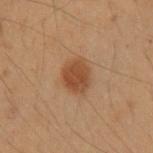<lesion>
<biopsy_status>not biopsied; imaged during a skin examination</biopsy_status>
<lighting>cross-polarized</lighting>
<automated_metrics>
  <area_mm2_approx>9.0</area_mm2_approx>
  <shape_asymmetry>0.15</shape_asymmetry>
  <cielab_L>38</cielab_L>
  <cielab_a>18</cielab_a>
  <cielab_b>29</cielab_b>
  <vs_skin_darker_L>9.0</vs_skin_darker_L>
  <vs_skin_contrast_norm>8.0</vs_skin_contrast_norm>
</automated_metrics>
<image>
  <source>total-body photography crop</source>
  <field_of_view_mm>15</field_of_view_mm>
</image>
<site>right forearm</site>
<patient>
  <sex>female</sex>
  <age_approx>40</age_approx>
</patient>
<lesion_size>
  <long_diameter_mm_approx>3.5</long_diameter_mm_approx>
</lesion_size>
</lesion>